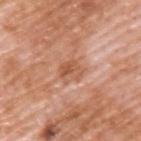Captured during whole-body skin photography for melanoma surveillance; the lesion was not biopsied. This is a white-light tile. Located on the upper back. A roughly 15 mm field-of-view crop from a total-body skin photograph. Automated image analysis of the tile measured a mean CIELAB color near L≈56 a*≈25 b*≈34, about 9 CIELAB-L* units darker than the surrounding skin, and a normalized border contrast of about 6.5. The analysis additionally found a border-irregularity index near 3.5/10. The software also gave an automated nevus-likeness rating near 0 out of 100 and lesion-presence confidence of about 100/100. Measured at roughly 3 mm in maximum diameter. The subject is a male aged 58–62.